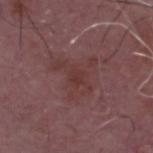automated_metrics:
  color_variation_0_10: 2.5
  peripheral_color_asymmetry: 0.5
site: head or neck
patient:
  sex: male
  age_approx: 65
image:
  source: total-body photography crop
  field_of_view_mm: 15
lighting: white-light